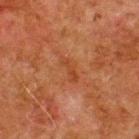Notes:
- workup — total-body-photography surveillance lesion; no biopsy
- body site — the upper back
- illumination — cross-polarized
- lesion size — ≈3.5 mm
- patient — male, aged approximately 80
- acquisition — ~15 mm crop, total-body skin-cancer survey
- TBP lesion metrics — about 5 CIELAB-L* units darker than the surrounding skin and a normalized lesion–skin contrast near 5; border irregularity of about 4 on a 0–10 scale, a color-variation rating of about 0.5/10, and radial color variation of about 0; an automated nevus-likeness rating near 0 out of 100 and a detector confidence of about 100 out of 100 that the crop contains a lesion